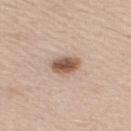biopsy status — total-body-photography surveillance lesion; no biopsy
lesion size — ≈3.5 mm
acquisition — ~15 mm tile from a whole-body skin photo
lighting — white-light
body site — the upper back
automated lesion analysis — a lesion area of about 6.5 mm² and an eccentricity of roughly 0.75; a mean CIELAB color near L≈56 a*≈19 b*≈28, roughly 15 lightness units darker than nearby skin, and a normalized lesion–skin contrast near 10; border irregularity of about 1.5 on a 0–10 scale, a within-lesion color-variation index near 5.5/10, and a peripheral color-asymmetry measure near 1.5; an automated nevus-likeness rating near 100 out of 100 and a detector confidence of about 100 out of 100 that the crop contains a lesion
patient — male, roughly 45 years of age